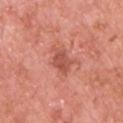This lesion was catalogued during total-body skin photography and was not selected for biopsy. Longest diameter approximately 3.5 mm. The lesion is on the chest. This is a white-light tile. A male patient aged 68–72. A region of skin cropped from a whole-body photographic capture, roughly 15 mm wide.